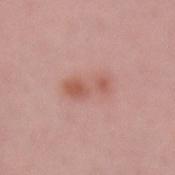The recorded lesion diameter is about 4.5 mm. Captured under white-light illumination. Located on the left forearm. A female patient, aged around 45. Cropped from a total-body skin-imaging series; the visible field is about 15 mm. Automated image analysis of the tile measured a shape eccentricity near 0.9 and two-axis asymmetry of about 0.4. The analysis additionally found peripheral color asymmetry of about 1. The analysis additionally found an automated nevus-likeness rating near 20 out of 100 and a detector confidence of about 100 out of 100 that the crop contains a lesion.